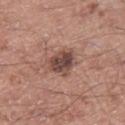Case summary:
– notes · no biopsy performed (imaged during a skin exam)
– anatomic site · the right thigh
– image · total-body-photography crop, ~15 mm field of view
– tile lighting · white-light
– subject · male, roughly 55 years of age